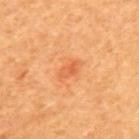workup: total-body-photography surveillance lesion; no biopsy | location: the back | illumination: cross-polarized | lesion size: about 2.5 mm | subject: female, aged approximately 40 | TBP lesion metrics: an average lesion color of about L≈63 a*≈33 b*≈45 (CIELAB), roughly 8 lightness units darker than nearby skin, and a normalized lesion–skin contrast near 5; a border-irregularity index near 2/10 and a peripheral color-asymmetry measure near 1.5; an automated nevus-likeness rating near 5 out of 100 | acquisition: 15 mm crop, total-body photography.